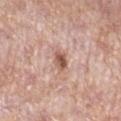<case>
  <lesion_size>
    <long_diameter_mm_approx>2.5</long_diameter_mm_approx>
  </lesion_size>
  <site>right lower leg</site>
  <lighting>white-light</lighting>
  <patient>
    <sex>female</sex>
    <age_approx>70</age_approx>
  </patient>
  <automated_metrics>
    <area_mm2_approx>4.0</area_mm2_approx>
    <eccentricity>0.7</eccentricity>
    <shape_asymmetry>0.25</shape_asymmetry>
    <cielab_L>56</cielab_L>
    <cielab_a>21</cielab_a>
    <cielab_b>28</cielab_b>
    <vs_skin_darker_L>13.0</vs_skin_darker_L>
    <vs_skin_contrast_norm>8.5</vs_skin_contrast_norm>
    <border_irregularity_0_10>2.0</border_irregularity_0_10>
  </automated_metrics>
  <image>
    <source>total-body photography crop</source>
    <field_of_view_mm>15</field_of_view_mm>
  </image>
</case>